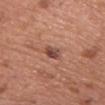Q: Was this lesion biopsied?
A: imaged on a skin check; not biopsied
Q: What is the imaging modality?
A: ~15 mm tile from a whole-body skin photo
Q: Lesion location?
A: the chest
Q: What are the patient's age and sex?
A: female, approximately 60 years of age